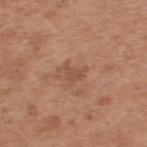Findings:
– notes · imaged on a skin check; not biopsied
– patient · male, aged approximately 55
– size · about 2.5 mm
– acquisition · total-body-photography crop, ~15 mm field of view
– image-analysis metrics · a footprint of about 3.5 mm², an eccentricity of roughly 0.7, and a symmetry-axis asymmetry near 0.5
– illumination · white-light
– location · the back